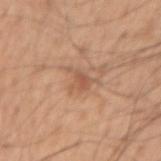Assessment: Part of a total-body skin-imaging series; this lesion was reviewed on a skin check and was not flagged for biopsy. Acquisition and patient details: Captured under white-light illumination. The total-body-photography lesion software estimated an average lesion color of about L≈56 a*≈21 b*≈33 (CIELAB) and a normalized lesion–skin contrast near 5.5. A male patient, aged approximately 55. Cropped from a total-body skin-imaging series; the visible field is about 15 mm. Longest diameter approximately 3.5 mm. From the right upper arm.